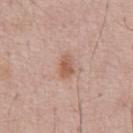Q: How was this image acquired?
A: total-body-photography crop, ~15 mm field of view
Q: How was the tile lit?
A: white-light illumination
Q: Who is the patient?
A: male, approximately 55 years of age
Q: How large is the lesion?
A: about 2.5 mm